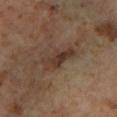Recorded during total-body skin imaging; not selected for excision or biopsy. Automated image analysis of the tile measured a symmetry-axis asymmetry near 0.35. The analysis additionally found a mean CIELAB color near L≈33 a*≈16 b*≈24, roughly 9 lightness units darker than nearby skin, and a normalized border contrast of about 8.5. And it measured a border-irregularity rating of about 4.5/10, internal color variation of about 4 on a 0–10 scale, and peripheral color asymmetry of about 1.5. The tile uses cross-polarized illumination. A 15 mm close-up tile from a total-body photography series done for melanoma screening. The recorded lesion diameter is about 4.5 mm. On the left lower leg. A male patient, approximately 60 years of age.